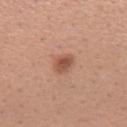Captured during whole-body skin photography for melanoma surveillance; the lesion was not biopsied. Approximately 2.5 mm at its widest. Imaged with white-light lighting. The lesion is located on the left forearm. A female subject, in their 30s. This image is a 15 mm lesion crop taken from a total-body photograph. The lesion-visualizer software estimated a footprint of about 4.5 mm², an outline eccentricity of about 0.65 (0 = round, 1 = elongated), and a symmetry-axis asymmetry near 0.2. The analysis additionally found a classifier nevus-likeness of about 90/100 and lesion-presence confidence of about 100/100.